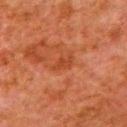notes: imaged on a skin check; not biopsied | automated metrics: a border-irregularity rating of about 3.5/10, a color-variation rating of about 0.5/10, and a peripheral color-asymmetry measure near 0; an automated nevus-likeness rating near 0 out of 100 and a lesion-detection confidence of about 100/100 | imaging modality: ~15 mm crop, total-body skin-cancer survey | tile lighting: cross-polarized | lesion diameter: ≈3 mm | site: the left upper arm | patient: male, aged around 80.